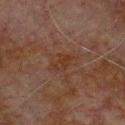image: total-body-photography crop, ~15 mm field of view
anatomic site: the chest
illumination: cross-polarized
TBP lesion metrics: a lesion area of about 4.5 mm², an eccentricity of roughly 0.8, and a symmetry-axis asymmetry near 0.4; a border-irregularity index near 4.5/10 and a color-variation rating of about 3.5/10
lesion size: ~3 mm (longest diameter)
subject: male, roughly 80 years of age